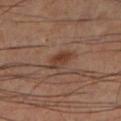<lesion>
<biopsy_status>not biopsied; imaged during a skin examination</biopsy_status>
<image>
  <source>total-body photography crop</source>
  <field_of_view_mm>15</field_of_view_mm>
</image>
<site>left lower leg</site>
<lighting>cross-polarized</lighting>
<patient>
  <sex>male</sex>
  <age_approx>60</age_approx>
</patient>
</lesion>